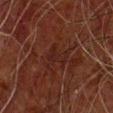Captured during whole-body skin photography for melanoma surveillance; the lesion was not biopsied. Imaged with cross-polarized lighting. On the chest. Longest diameter approximately 3.5 mm. The patient is a male aged 58 to 62. Automated image analysis of the tile measured a normalized lesion–skin contrast near 4.5. A region of skin cropped from a whole-body photographic capture, roughly 15 mm wide.